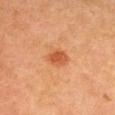Longest diameter approximately 3 mm. A 15 mm close-up tile from a total-body photography series done for melanoma screening. The subject is a female aged 18 to 22. An algorithmic analysis of the crop reported a border-irregularity index near 2/10 and a within-lesion color-variation index near 3/10. The analysis additionally found a classifier nevus-likeness of about 95/100 and lesion-presence confidence of about 100/100. The lesion is on the head or neck. The tile uses cross-polarized illumination.